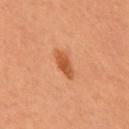The lesion was tiled from a total-body skin photograph and was not biopsied. This is a cross-polarized tile. The lesion-visualizer software estimated a border-irregularity index near 2.5/10, a within-lesion color-variation index near 3.5/10, and a peripheral color-asymmetry measure near 1. And it measured a classifier nevus-likeness of about 95/100. A female subject aged approximately 50. A 15 mm crop from a total-body photograph taken for skin-cancer surveillance.